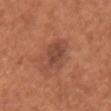No biopsy was performed on this lesion — it was imaged during a full skin examination and was not determined to be concerning. On the front of the torso. Cropped from a total-body skin-imaging series; the visible field is about 15 mm. A female subject, approximately 50 years of age. The lesion's longest dimension is about 4 mm. The tile uses white-light illumination.